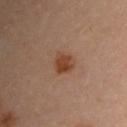* follow-up — catalogued during a skin exam; not biopsied
* image source — ~15 mm crop, total-body skin-cancer survey
* lesion diameter — ~3 mm (longest diameter)
* anatomic site — the left upper arm
* patient — female, about 30 years old
* lighting — cross-polarized illumination